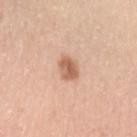Clinical impression: Recorded during total-body skin imaging; not selected for excision or biopsy. Image and clinical context: On the left upper arm. The tile uses white-light illumination. About 2.5 mm across. The subject is a female approximately 40 years of age. A close-up tile cropped from a whole-body skin photograph, about 15 mm across.